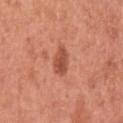<record>
  <biopsy_status>not biopsied; imaged during a skin examination</biopsy_status>
  <patient>
    <sex>female</sex>
    <age_approx>50</age_approx>
  </patient>
  <image>
    <source>total-body photography crop</source>
    <field_of_view_mm>15</field_of_view_mm>
  </image>
  <automated_metrics>
    <area_mm2_approx>5.5</area_mm2_approx>
    <eccentricity>0.85</eccentricity>
    <border_irregularity_0_10>2.5</border_irregularity_0_10>
    <color_variation_0_10>2.5</color_variation_0_10>
    <lesion_detection_confidence_0_100>100</lesion_detection_confidence_0_100>
  </automated_metrics>
  <site>left upper arm</site>
</record>